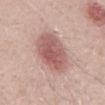Part of a total-body skin-imaging series; this lesion was reviewed on a skin check and was not flagged for biopsy.